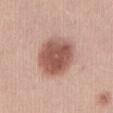The lesion was tiled from a total-body skin photograph and was not biopsied. Approximately 5.5 mm at its widest. Located on the front of the torso. A female patient, aged around 30. An algorithmic analysis of the crop reported an area of roughly 21 mm², a shape eccentricity near 0.4, and two-axis asymmetry of about 0.1. The software also gave a lesion color around L≈55 a*≈23 b*≈26 in CIELAB and a normalized lesion–skin contrast near 9.5. And it measured a nevus-likeness score of about 95/100 and a lesion-detection confidence of about 100/100. This image is a 15 mm lesion crop taken from a total-body photograph. Imaged with white-light lighting.